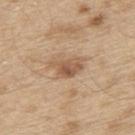Q: Was a biopsy performed?
A: no biopsy performed (imaged during a skin exam)
Q: Lesion size?
A: about 3.5 mm
Q: Where on the body is the lesion?
A: the upper back
Q: Patient demographics?
A: male, aged 68 to 72
Q: What kind of image is this?
A: ~15 mm tile from a whole-body skin photo
Q: How was the tile lit?
A: white-light